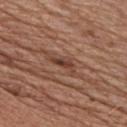The lesion was tiled from a total-body skin photograph and was not biopsied.
The lesion is located on the chest.
Captured under white-light illumination.
The lesion's longest dimension is about 4 mm.
An algorithmic analysis of the crop reported a mean CIELAB color near L≈42 a*≈22 b*≈27, roughly 9 lightness units darker than nearby skin, and a normalized lesion–skin contrast near 7.5. The analysis additionally found border irregularity of about 5 on a 0–10 scale, a color-variation rating of about 3.5/10, and peripheral color asymmetry of about 1. The analysis additionally found an automated nevus-likeness rating near 35 out of 100.
A male patient, aged 53 to 57.
A region of skin cropped from a whole-body photographic capture, roughly 15 mm wide.